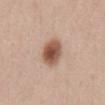Assessment: The lesion was photographed on a routine skin check and not biopsied; there is no pathology result. Context: A female patient about 35 years old. The lesion is located on the abdomen. A 15 mm crop from a total-body photograph taken for skin-cancer surveillance.